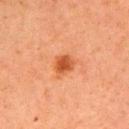Part of a total-body skin-imaging series; this lesion was reviewed on a skin check and was not flagged for biopsy. The lesion is on the left upper arm. Imaged with cross-polarized lighting. A lesion tile, about 15 mm wide, cut from a 3D total-body photograph. About 3 mm across. A female patient, aged around 60. Automated image analysis of the tile measured a border-irregularity rating of about 2.5/10, internal color variation of about 2.5 on a 0–10 scale, and peripheral color asymmetry of about 0.5.